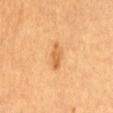Imaged during a routine full-body skin examination; the lesion was not biopsied and no histopathology is available. The patient is a male aged approximately 60. A 15 mm close-up tile from a total-body photography series done for melanoma screening. From the front of the torso. Imaged with cross-polarized lighting.